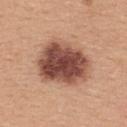Case summary:
• follow-up — catalogued during a skin exam; not biopsied
• image — total-body-photography crop, ~15 mm field of view
• site — the upper back
• patient — female, about 45 years old
• image-analysis metrics — a footprint of about 27 mm² and a symmetry-axis asymmetry near 0.2; a lesion color around L≈49 a*≈23 b*≈27 in CIELAB, a lesion–skin lightness drop of about 18, and a normalized lesion–skin contrast near 12.5; an automated nevus-likeness rating near 35 out of 100
• diameter — ≈6.5 mm